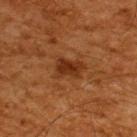Recorded during total-body skin imaging; not selected for excision or biopsy. The subject is a male roughly 65 years of age. The lesion is located on the upper back. Automated tile analysis of the lesion measured an automated nevus-likeness rating near 30 out of 100 and a lesion-detection confidence of about 100/100. A region of skin cropped from a whole-body photographic capture, roughly 15 mm wide.